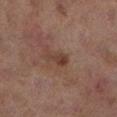Imaged during a routine full-body skin examination; the lesion was not biopsied and no histopathology is available. From the left lower leg. The lesion's longest dimension is about 3 mm. An algorithmic analysis of the crop reported an area of roughly 4 mm², an outline eccentricity of about 0.85 (0 = round, 1 = elongated), and two-axis asymmetry of about 0.4. The analysis additionally found a mean CIELAB color near L≈33 a*≈16 b*≈23, roughly 7 lightness units darker than nearby skin, and a normalized lesion–skin contrast near 7. And it measured border irregularity of about 3.5 on a 0–10 scale, a color-variation rating of about 3/10, and a peripheral color-asymmetry measure near 0.5. Imaged with cross-polarized lighting. A 15 mm close-up tile from a total-body photography series done for melanoma screening. A female subject, about 60 years old.